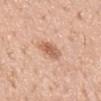Case summary:
* notes — imaged on a skin check; not biopsied
* image source — ~15 mm crop, total-body skin-cancer survey
* size — about 3.5 mm
* patient — female, aged approximately 50
* automated metrics — a mean CIELAB color near L≈62 a*≈22 b*≈33, about 11 CIELAB-L* units darker than the surrounding skin, and a lesion-to-skin contrast of about 7 (normalized; higher = more distinct)
* anatomic site — the mid back
* tile lighting — white-light